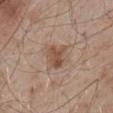Cropped from a whole-body photographic skin survey; the tile spans about 15 mm.
The tile uses white-light illumination.
Longest diameter approximately 3 mm.
A male subject, aged approximately 55.
The lesion is located on the mid back.